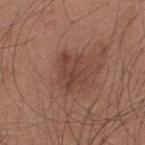{
  "biopsy_status": "not biopsied; imaged during a skin examination",
  "lesion_size": {
    "long_diameter_mm_approx": 4.0
  },
  "lighting": "white-light",
  "patient": {
    "sex": "male",
    "age_approx": 35
  },
  "automated_metrics": {
    "cielab_L": 42,
    "cielab_a": 22,
    "cielab_b": 26,
    "vs_skin_darker_L": 7.0,
    "vs_skin_contrast_norm": 6.0,
    "border_irregularity_0_10": 4.5,
    "peripheral_color_asymmetry": 1.5,
    "nevus_likeness_0_100": 0,
    "lesion_detection_confidence_0_100": 100
  },
  "image": {
    "source": "total-body photography crop",
    "field_of_view_mm": 15
  },
  "site": "back"
}